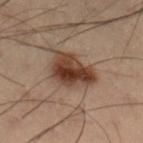| feature | finding |
|---|---|
| workup | catalogued during a skin exam; not biopsied |
| imaging modality | ~15 mm crop, total-body skin-cancer survey |
| lighting | cross-polarized illumination |
| diameter | about 5 mm |
| location | the right lower leg |
| patient | male, in their mid-50s |